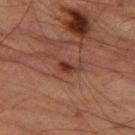* biopsy status: total-body-photography surveillance lesion; no biopsy
* image: total-body-photography crop, ~15 mm field of view
* size: ≈2.5 mm
* anatomic site: the left thigh
* automated lesion analysis: border irregularity of about 4 on a 0–10 scale and peripheral color asymmetry of about 0
* subject: male, roughly 85 years of age or older